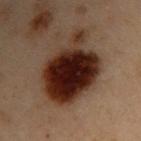  lighting: cross-polarized
  image:
    source: total-body photography crop
    field_of_view_mm: 15
  site: right upper arm
  lesion_size:
    long_diameter_mm_approx: 7.5
  patient:
    sex: male
    age_approx: 55